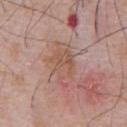  biopsy_status: not biopsied; imaged during a skin examination
  site: abdomen
  image:
    source: total-body photography crop
    field_of_view_mm: 15
  lighting: white-light
  patient:
    sex: male
    age_approx: 60
  lesion_size:
    long_diameter_mm_approx: 5.0
  automated_metrics:
    cielab_L: 54
    cielab_a: 20
    cielab_b: 27
    vs_skin_contrast_norm: 6.0
    peripheral_color_asymmetry: 1.5